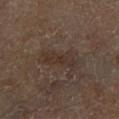The lesion was photographed on a routine skin check and not biopsied; there is no pathology result. A male subject roughly 70 years of age. Cropped from a whole-body photographic skin survey; the tile spans about 15 mm. On the right lower leg. This is a cross-polarized tile. Measured at roughly 4.5 mm in maximum diameter.